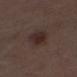Imaged during a routine full-body skin examination; the lesion was not biopsied and no histopathology is available.
Cropped from a whole-body photographic skin survey; the tile spans about 15 mm.
The lesion is located on the left lower leg.
The patient is a male aged 68 to 72.
The tile uses white-light illumination.
Longest diameter approximately 3.5 mm.
An algorithmic analysis of the crop reported a border-irregularity rating of about 1.5/10 and a peripheral color-asymmetry measure near 1.